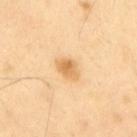| feature | finding |
|---|---|
| location | the upper back |
| image source | ~15 mm crop, total-body skin-cancer survey |
| subject | male, about 40 years old |